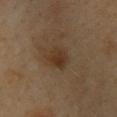{
  "biopsy_status": "not biopsied; imaged during a skin examination",
  "site": "upper back",
  "patient": {
    "sex": "male",
    "age_approx": 40
  },
  "image": {
    "source": "total-body photography crop",
    "field_of_view_mm": 15
  },
  "lighting": "cross-polarized"
}